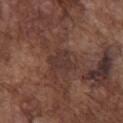Part of a total-body skin-imaging series; this lesion was reviewed on a skin check and was not flagged for biopsy. A close-up tile cropped from a whole-body skin photograph, about 15 mm across. A male patient about 75 years old. On the chest.